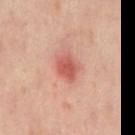Findings:
– biopsy status — imaged on a skin check; not biopsied
– imaging modality — 15 mm crop, total-body photography
– patient — male, aged 43–47
– location — the back